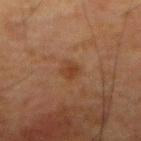notes = catalogued during a skin exam; not biopsied | body site = the abdomen | patient = male, approximately 80 years of age | lighting = cross-polarized | lesion diameter = about 2.5 mm | image source = ~15 mm crop, total-body skin-cancer survey.